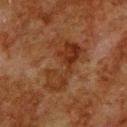Captured during whole-body skin photography for melanoma surveillance; the lesion was not biopsied. Imaged with cross-polarized lighting. On the back. A region of skin cropped from a whole-body photographic capture, roughly 15 mm wide. Longest diameter approximately 7 mm. A male subject approximately 80 years of age.